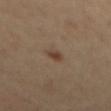Clinical impression:
This lesion was catalogued during total-body skin photography and was not selected for biopsy.
Clinical summary:
From the mid back. Measured at roughly 2 mm in maximum diameter. This image is a 15 mm lesion crop taken from a total-body photograph. A male patient, roughly 55 years of age.